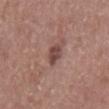Case summary:
• anatomic site: the chest
• TBP lesion metrics: a lesion area of about 4.5 mm²; a border-irregularity rating of about 2.5/10, internal color variation of about 2 on a 0–10 scale, and a peripheral color-asymmetry measure near 0.5; a lesion-detection confidence of about 100/100
• acquisition: total-body-photography crop, ~15 mm field of view
• patient: male, approximately 60 years of age
• lighting: white-light illumination